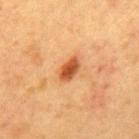• body site · the back
• image · 15 mm crop, total-body photography
• patient · female, aged 38–42
• automated metrics · a border-irregularity rating of about 2/10, internal color variation of about 3 on a 0–10 scale, and radial color variation of about 1
• lesion size · about 3 mm
• illumination · cross-polarized